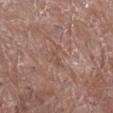Impression:
Recorded during total-body skin imaging; not selected for excision or biopsy.
Image and clinical context:
Imaged with white-light lighting. A female patient aged approximately 80. The lesion is on the right lower leg. This image is a 15 mm lesion crop taken from a total-body photograph. The recorded lesion diameter is about 3.5 mm.